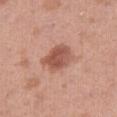notes: total-body-photography surveillance lesion; no biopsy
patient: female, aged around 40
anatomic site: the right thigh
acquisition: ~15 mm crop, total-body skin-cancer survey